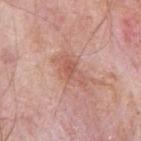| field | value |
|---|---|
| biopsy status | no biopsy performed (imaged during a skin exam) |
| patient | male, aged approximately 70 |
| imaging modality | 15 mm crop, total-body photography |
| anatomic site | the right upper arm |